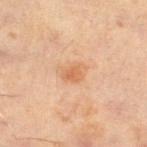Captured during whole-body skin photography for melanoma surveillance; the lesion was not biopsied.
A region of skin cropped from a whole-body photographic capture, roughly 15 mm wide.
The lesion is on the right thigh.
A male patient, roughly 60 years of age.
The tile uses cross-polarized illumination.
Automated tile analysis of the lesion measured an average lesion color of about L≈60 a*≈22 b*≈36 (CIELAB) and a lesion–skin lightness drop of about 8. And it measured a nevus-likeness score of about 20/100.
Approximately 3 mm at its widest.